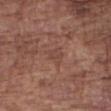<lesion>
  <biopsy_status>not biopsied; imaged during a skin examination</biopsy_status>
</lesion>